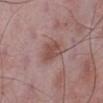  biopsy_status: not biopsied; imaged during a skin examination
  site: left lower leg
  lesion_size:
    long_diameter_mm_approx: 3.5
  patient:
    sex: male
    age_approx: 50
  image:
    source: total-body photography crop
    field_of_view_mm: 15